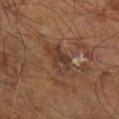notes: imaged on a skin check; not biopsied | acquisition: ~15 mm crop, total-body skin-cancer survey | patient: male, aged 58 to 62 | site: the right leg | lighting: cross-polarized.